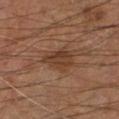Case summary:
- biopsy status · imaged on a skin check; not biopsied
- automated metrics · a lesion-to-skin contrast of about 7 (normalized; higher = more distinct)
- site · the leg
- imaging modality · 15 mm crop, total-body photography
- subject · male, approximately 60 years of age
- lighting · cross-polarized
- lesion diameter · about 4 mm